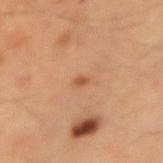Impression:
Imaged during a routine full-body skin examination; the lesion was not biopsied and no histopathology is available.
Context:
A lesion tile, about 15 mm wide, cut from a 3D total-body photograph. On the right forearm. A male patient aged around 60. Captured under cross-polarized illumination.